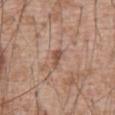Q: Is there a histopathology result?
A: imaged on a skin check; not biopsied
Q: How was the tile lit?
A: white-light
Q: What kind of image is this?
A: ~15 mm tile from a whole-body skin photo
Q: Patient demographics?
A: male, aged around 60
Q: What is the anatomic site?
A: the abdomen